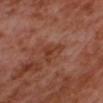| key | value |
|---|---|
| notes | no biopsy performed (imaged during a skin exam) |
| automated lesion analysis | a lesion area of about 5 mm²; a normalized border contrast of about 6.5 |
| subject | male, in their mid-50s |
| lesion diameter | ~3.5 mm (longest diameter) |
| illumination | cross-polarized |
| anatomic site | the head or neck |
| image | ~15 mm tile from a whole-body skin photo |